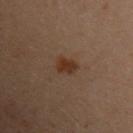notes=no biopsy performed (imaged during a skin exam)
image=total-body-photography crop, ~15 mm field of view
anatomic site=the left arm
patient=female, aged around 30